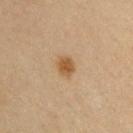Clinical impression:
The lesion was tiled from a total-body skin photograph and was not biopsied.
Clinical summary:
Imaged with cross-polarized lighting. The patient is a male in their mid- to late 30s. Approximately 2.5 mm at its widest. Located on the back. A region of skin cropped from a whole-body photographic capture, roughly 15 mm wide.